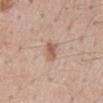Clinical impression:
The lesion was tiled from a total-body skin photograph and was not biopsied.
Image and clinical context:
The subject is a male aged around 60. A 15 mm close-up tile from a total-body photography series done for melanoma screening. The lesion's longest dimension is about 3.5 mm. The lesion is located on the mid back.